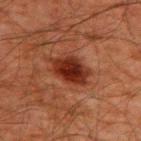Assessment:
The lesion was tiled from a total-body skin photograph and was not biopsied.
Background:
A 15 mm crop from a total-body photograph taken for skin-cancer surveillance. The lesion is located on the upper back. An algorithmic analysis of the crop reported a shape eccentricity near 0.75 and a shape-asymmetry score of about 0.2 (0 = symmetric). It also reported an average lesion color of about L≈23 a*≈24 b*≈25 (CIELAB), a lesion–skin lightness drop of about 12, and a normalized border contrast of about 12. It also reported a border-irregularity index near 2/10, internal color variation of about 4 on a 0–10 scale, and peripheral color asymmetry of about 1. A male patient approximately 60 years of age.